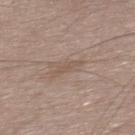Imaged during a routine full-body skin examination; the lesion was not biopsied and no histopathology is available.
The lesion is located on the mid back.
A 15 mm close-up tile from a total-body photography series done for melanoma screening.
Imaged with white-light lighting.
A male subject, aged 53–57.